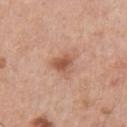workup = imaged on a skin check; not biopsied
subject = male, aged 58–62
acquisition = total-body-photography crop, ~15 mm field of view
lesion size = about 2.5 mm
image-analysis metrics = an eccentricity of roughly 0.5 and two-axis asymmetry of about 0.4; a lesion–skin lightness drop of about 11 and a normalized lesion–skin contrast near 7.5; an automated nevus-likeness rating near 50 out of 100
lighting = white-light
body site = the front of the torso